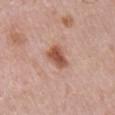No biopsy was performed on this lesion — it was imaged during a full skin examination and was not determined to be concerning. A 15 mm close-up tile from a total-body photography series done for melanoma screening. The lesion-visualizer software estimated a lesion color around L≈54 a*≈24 b*≈30 in CIELAB, a lesion–skin lightness drop of about 13, and a normalized border contrast of about 9.5. The tile uses white-light illumination. The lesion is on the chest. A male patient aged around 80.